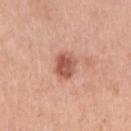| key | value |
|---|---|
| notes | imaged on a skin check; not biopsied |
| body site | the right upper arm |
| lighting | white-light illumination |
| image source | 15 mm crop, total-body photography |
| patient | female, roughly 60 years of age |